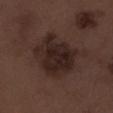The lesion was photographed on a routine skin check and not biopsied; there is no pathology result. Captured under white-light illumination. Located on the right lower leg. A male patient, aged around 70. A close-up tile cropped from a whole-body skin photograph, about 15 mm across.